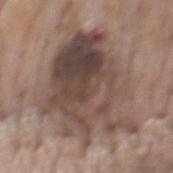{"biopsy_status": "not biopsied; imaged during a skin examination", "image": {"source": "total-body photography crop", "field_of_view_mm": 15}, "lighting": "white-light", "automated_metrics": {"eccentricity": 0.65, "shape_asymmetry": 0.3, "nevus_likeness_0_100": 0}, "patient": {"sex": "male", "age_approx": 75}, "lesion_size": {"long_diameter_mm_approx": 11.0}, "site": "mid back"}